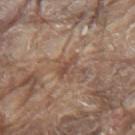biopsy status — no biopsy performed (imaged during a skin exam) | lesion diameter — ~3 mm (longest diameter) | site — the back | imaging modality — 15 mm crop, total-body photography | lighting — white-light | subject — male, about 80 years old | TBP lesion metrics — a shape eccentricity near 0.85 and two-axis asymmetry of about 0.35; border irregularity of about 3.5 on a 0–10 scale and a color-variation rating of about 3/10; an automated nevus-likeness rating near 0 out of 100 and lesion-presence confidence of about 50/100.